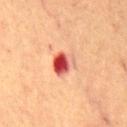Notes:
* biopsy status: total-body-photography surveillance lesion; no biopsy
* subject: male, roughly 65 years of age
* site: the back
* image source: 15 mm crop, total-body photography
* illumination: cross-polarized
* diameter: ~3 mm (longest diameter)